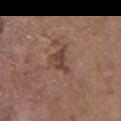Notes:
* image — ~15 mm crop, total-body skin-cancer survey
* illumination — white-light illumination
* location — the chest
* lesion diameter — ~4 mm (longest diameter)
* automated metrics — a mean CIELAB color near L≈42 a*≈19 b*≈24, about 9 CIELAB-L* units darker than the surrounding skin, and a lesion-to-skin contrast of about 7.5 (normalized; higher = more distinct); a border-irregularity rating of about 5.5/10, a within-lesion color-variation index near 2.5/10, and peripheral color asymmetry of about 1
* patient — female, aged approximately 65